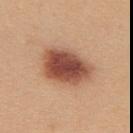Clinical impression: The lesion was tiled from a total-body skin photograph and was not biopsied. Context: Longest diameter approximately 6 mm. A female subject in their mid- to late 20s. The total-body-photography lesion software estimated a footprint of about 20 mm² and a symmetry-axis asymmetry near 0.2. It also reported a classifier nevus-likeness of about 100/100. A 15 mm close-up tile from a total-body photography series done for melanoma screening. Imaged with white-light lighting. Located on the upper back.